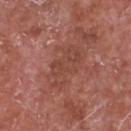The lesion was photographed on a routine skin check and not biopsied; there is no pathology result. The tile uses white-light illumination. A close-up tile cropped from a whole-body skin photograph, about 15 mm across. The total-body-photography lesion software estimated an automated nevus-likeness rating near 0 out of 100 and a lesion-detection confidence of about 100/100. From the chest. Longest diameter approximately 5.5 mm. A male subject, aged around 65.